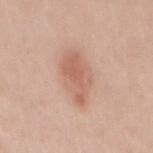workup: no biopsy performed (imaged during a skin exam)
image-analysis metrics: a footprint of about 13 mm², an eccentricity of roughly 0.85, and a shape-asymmetry score of about 0.2 (0 = symmetric); internal color variation of about 3 on a 0–10 scale and a peripheral color-asymmetry measure near 1; a lesion-detection confidence of about 100/100
lighting: white-light illumination
site: the mid back
patient: male, aged approximately 35
image: ~15 mm tile from a whole-body skin photo